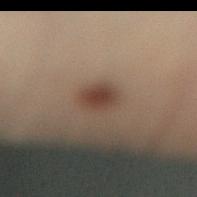Recorded during total-body skin imaging; not selected for excision or biopsy. Measured at roughly 3 mm in maximum diameter. A male patient approximately 50 years of age. Cropped from a whole-body photographic skin survey; the tile spans about 15 mm. The lesion is on the left leg.